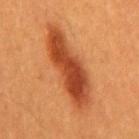notes = catalogued during a skin exam; not biopsied | patient = male, about 60 years old | automated lesion analysis = a mean CIELAB color near L≈45 a*≈29 b*≈39, about 13 CIELAB-L* units darker than the surrounding skin, and a normalized lesion–skin contrast near 9.5; a classifier nevus-likeness of about 95/100 | illumination = cross-polarized | imaging modality = total-body-photography crop, ~15 mm field of view | diameter = ≈10.5 mm | body site = the mid back.